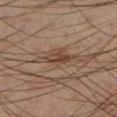The lesion was tiled from a total-body skin photograph and was not biopsied. This image is a 15 mm lesion crop taken from a total-body photograph. The patient is a male aged 43 to 47. The lesion is located on the left lower leg. The tile uses cross-polarized illumination. The recorded lesion diameter is about 3 mm. The lesion-visualizer software estimated an area of roughly 4 mm², an eccentricity of roughly 0.9, and a shape-asymmetry score of about 0.25 (0 = symmetric). The software also gave a border-irregularity index near 3/10, a within-lesion color-variation index near 3/10, and peripheral color asymmetry of about 1.